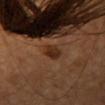notes: catalogued during a skin exam; not biopsied
location: the head or neck
image: total-body-photography crop, ~15 mm field of view
subject: female, aged 38 to 42